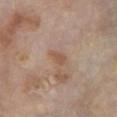The lesion was tiled from a total-body skin photograph and was not biopsied.